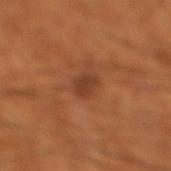Clinical impression:
Captured during whole-body skin photography for melanoma surveillance; the lesion was not biopsied.
Acquisition and patient details:
The tile uses cross-polarized illumination. The lesion is on the left leg. The subject is a male aged 63 to 67. A roughly 15 mm field-of-view crop from a total-body skin photograph. Longest diameter approximately 2.5 mm.